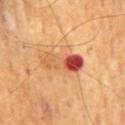Clinical impression: Part of a total-body skin-imaging series; this lesion was reviewed on a skin check and was not flagged for biopsy. Context: On the lower back. A region of skin cropped from a whole-body photographic capture, roughly 15 mm wide. The recorded lesion diameter is about 5.5 mm. A male subject aged around 70. The tile uses cross-polarized illumination.